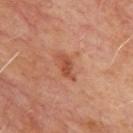Q: Was this lesion biopsied?
A: catalogued during a skin exam; not biopsied
Q: Automated lesion metrics?
A: a lesion color around L≈48 a*≈27 b*≈32 in CIELAB, roughly 9 lightness units darker than nearby skin, and a normalized border contrast of about 7; a border-irregularity rating of about 3.5/10, a within-lesion color-variation index near 1.5/10, and peripheral color asymmetry of about 0.5; a classifier nevus-likeness of about 45/100 and lesion-presence confidence of about 100/100
Q: Who is the patient?
A: male, approximately 65 years of age
Q: What is the lesion's diameter?
A: about 3.5 mm
Q: What lighting was used for the tile?
A: cross-polarized illumination
Q: What is the imaging modality?
A: ~15 mm tile from a whole-body skin photo
Q: What is the anatomic site?
A: the upper back